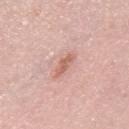Recorded during total-body skin imaging; not selected for excision or biopsy.
Located on the back.
A male subject approximately 55 years of age.
A roughly 15 mm field-of-view crop from a total-body skin photograph.